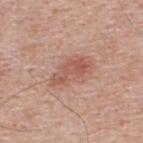Q: Was a biopsy performed?
A: imaged on a skin check; not biopsied
Q: What lighting was used for the tile?
A: white-light illumination
Q: How large is the lesion?
A: about 5 mm
Q: Lesion location?
A: the upper back
Q: What did automated image analysis measure?
A: a lesion area of about 10 mm², a shape eccentricity near 0.9, and a symmetry-axis asymmetry near 0.25; a within-lesion color-variation index near 4/10 and a peripheral color-asymmetry measure near 1.5
Q: What is the imaging modality?
A: total-body-photography crop, ~15 mm field of view
Q: Who is the patient?
A: male, aged 38–42